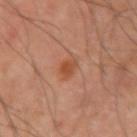biopsy_status: not biopsied; imaged during a skin examination
patient:
  sex: male
  age_approx: 40
image:
  source: total-body photography crop
  field_of_view_mm: 15
site: left upper arm